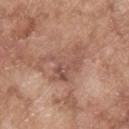Findings:
* notes — total-body-photography surveillance lesion; no biopsy
* diameter — about 6 mm
* illumination — white-light
* subject — male, about 65 years old
* automated metrics — a border-irregularity index near 8/10 and peripheral color asymmetry of about 1.5; an automated nevus-likeness rating near 0 out of 100 and a lesion-detection confidence of about 85/100
* anatomic site — the upper back
* image source — ~15 mm crop, total-body skin-cancer survey